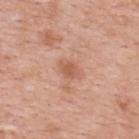Clinical impression: The lesion was tiled from a total-body skin photograph and was not biopsied. Context: A female subject, approximately 40 years of age. The lesion-visualizer software estimated a lesion area of about 3.5 mm² and an eccentricity of roughly 0.7. The analysis additionally found a color-variation rating of about 1.5/10 and a peripheral color-asymmetry measure near 0.5. It also reported a nevus-likeness score of about 20/100 and a lesion-detection confidence of about 100/100. A close-up tile cropped from a whole-body skin photograph, about 15 mm across. The lesion is located on the back. The tile uses white-light illumination.